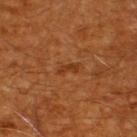No biopsy was performed on this lesion — it was imaged during a full skin examination and was not determined to be concerning.
This image is a 15 mm lesion crop taken from a total-body photograph.
A male subject, roughly 60 years of age.
Captured under cross-polarized illumination.
Approximately 2.5 mm at its widest.
Located on the back.